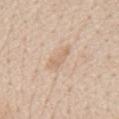Part of a total-body skin-imaging series; this lesion was reviewed on a skin check and was not flagged for biopsy.